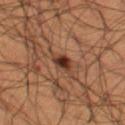Findings:
* notes — imaged on a skin check; not biopsied
* subject — male, aged approximately 50
* imaging modality — 15 mm crop, total-body photography
* tile lighting — cross-polarized illumination
* lesion size — ≈3 mm
* site — the left thigh
* image-analysis metrics — an automated nevus-likeness rating near 95 out of 100 and a detector confidence of about 100 out of 100 that the crop contains a lesion